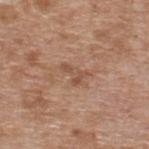Q: Was this lesion biopsied?
A: imaged on a skin check; not biopsied
Q: What is the anatomic site?
A: the back
Q: What did automated image analysis measure?
A: a lesion color around L≈51 a*≈20 b*≈30 in CIELAB, a lesion–skin lightness drop of about 8, and a normalized lesion–skin contrast near 6; a border-irregularity index near 7/10, a color-variation rating of about 0/10, and peripheral color asymmetry of about 0; an automated nevus-likeness rating near 0 out of 100
Q: Patient demographics?
A: male, roughly 60 years of age
Q: What kind of image is this?
A: total-body-photography crop, ~15 mm field of view